A close-up tile cropped from a whole-body skin photograph, about 15 mm across; a female patient, aged 58–62; from the left arm:
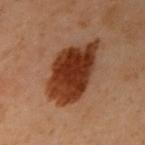Q: What lighting was used for the tile?
A: cross-polarized
Q: Automated lesion metrics?
A: an eccentricity of roughly 0.8 and two-axis asymmetry of about 0.25; border irregularity of about 3 on a 0–10 scale, a color-variation rating of about 4.5/10, and radial color variation of about 1.5; an automated nevus-likeness rating near 100 out of 100
Q: Lesion size?
A: about 8 mm
Q: What did the biopsy show?
A: a dysplastic (Clark) nevus — a benign skin lesion